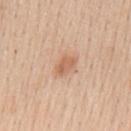No biopsy was performed on this lesion — it was imaged during a full skin examination and was not determined to be concerning. A male subject, aged 58–62. This is a white-light tile. The lesion-visualizer software estimated an area of roughly 4 mm², an outline eccentricity of about 0.8 (0 = round, 1 = elongated), and two-axis asymmetry of about 0.3. And it measured internal color variation of about 2.5 on a 0–10 scale and radial color variation of about 1. It also reported a nevus-likeness score of about 45/100 and a detector confidence of about 100 out of 100 that the crop contains a lesion. A region of skin cropped from a whole-body photographic capture, roughly 15 mm wide. From the mid back. About 3 mm across.